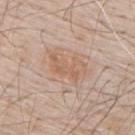{
  "biopsy_status": "not biopsied; imaged during a skin examination",
  "patient": {
    "sex": "male",
    "age_approx": 70
  },
  "lesion_size": {
    "long_diameter_mm_approx": 4.5
  },
  "image": {
    "source": "total-body photography crop",
    "field_of_view_mm": 15
  },
  "lighting": "white-light",
  "site": "upper back"
}